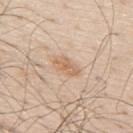image: ~15 mm crop, total-body skin-cancer survey | site: the upper back | subject: male, aged approximately 80 | lesion size: ≈3.5 mm.